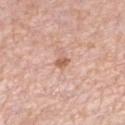Q: Was a biopsy performed?
A: total-body-photography surveillance lesion; no biopsy
Q: Who is the patient?
A: female, aged around 40
Q: What is the anatomic site?
A: the left lower leg
Q: Lesion size?
A: ≈1.5 mm
Q: How was this image acquired?
A: ~15 mm tile from a whole-body skin photo
Q: Automated lesion metrics?
A: a mean CIELAB color near L≈60 a*≈23 b*≈31, roughly 11 lightness units darker than nearby skin, and a lesion-to-skin contrast of about 7.5 (normalized; higher = more distinct); a border-irregularity index near 2.5/10, a color-variation rating of about 2/10, and radial color variation of about 1; a nevus-likeness score of about 20/100
Q: What lighting was used for the tile?
A: white-light